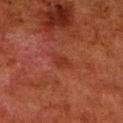<tbp_lesion>
<biopsy_status>not biopsied; imaged during a skin examination</biopsy_status>
<automated_metrics>
  <area_mm2_approx>3.5</area_mm2_approx>
  <eccentricity>0.8</eccentricity>
  <shape_asymmetry>0.3</shape_asymmetry>
  <cielab_L>28</cielab_L>
  <cielab_a>24</cielab_a>
  <cielab_b>27</cielab_b>
  <vs_skin_darker_L>5.0</vs_skin_darker_L>
  <vs_skin_contrast_norm>5.5</vs_skin_contrast_norm>
  <border_irregularity_0_10>3.0</border_irregularity_0_10>
  <peripheral_color_asymmetry>0.5</peripheral_color_asymmetry>
  <nevus_likeness_0_100>0</nevus_likeness_0_100>
  <lesion_detection_confidence_0_100>100</lesion_detection_confidence_0_100>
</automated_metrics>
<lighting>cross-polarized</lighting>
<site>right lower leg</site>
<lesion_size>
  <long_diameter_mm_approx>3.0</long_diameter_mm_approx>
</lesion_size>
<patient>
  <sex>male</sex>
  <age_approx>80</age_approx>
</patient>
<image>
  <source>total-body photography crop</source>
  <field_of_view_mm>15</field_of_view_mm>
</image>
</tbp_lesion>